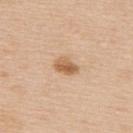Impression:
Imaged during a routine full-body skin examination; the lesion was not biopsied and no histopathology is available.
Context:
A 15 mm crop from a total-body photograph taken for skin-cancer surveillance. The tile uses white-light illumination. The subject is a female about 45 years old. The lesion is located on the upper back. The recorded lesion diameter is about 3 mm.